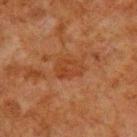| field | value |
|---|---|
| subject | male, aged 78 to 82 |
| image | ~15 mm crop, total-body skin-cancer survey |
| lesion size | about 3.5 mm |
| tile lighting | cross-polarized illumination |
| anatomic site | the upper back |